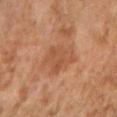  biopsy_status: not biopsied; imaged during a skin examination
  site: right upper arm
  image:
    source: total-body photography crop
    field_of_view_mm: 15
  patient:
    sex: female
    age_approx: 60